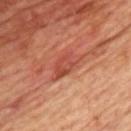Part of a total-body skin-imaging series; this lesion was reviewed on a skin check and was not flagged for biopsy. Approximately 4.5 mm at its widest. The subject is a male in their 70s. The lesion is located on the chest. Cropped from a total-body skin-imaging series; the visible field is about 15 mm. This is a cross-polarized tile.